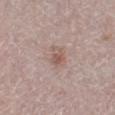Impression:
This lesion was catalogued during total-body skin photography and was not selected for biopsy.
Background:
This image is a 15 mm lesion crop taken from a total-body photograph. A female subject aged around 55. Located on the right lower leg. Longest diameter approximately 2.5 mm.